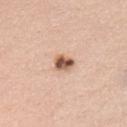Part of a total-body skin-imaging series; this lesion was reviewed on a skin check and was not flagged for biopsy. Located on the front of the torso. The subject is a male in their mid- to late 30s. Approximately 2.5 mm at its widest. Imaged with white-light lighting. Cropped from a whole-body photographic skin survey; the tile spans about 15 mm.